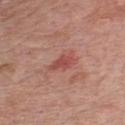Clinical impression:
Recorded during total-body skin imaging; not selected for excision or biopsy.